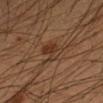Assessment:
Imaged during a routine full-body skin examination; the lesion was not biopsied and no histopathology is available.
Image and clinical context:
A roughly 15 mm field-of-view crop from a total-body skin photograph. The tile uses cross-polarized illumination. A male patient aged 33 to 37. The lesion is on the left forearm. The lesion-visualizer software estimated a footprint of about 6.5 mm² and a shape eccentricity near 0.75. The analysis additionally found a border-irregularity rating of about 3.5/10 and internal color variation of about 5 on a 0–10 scale.